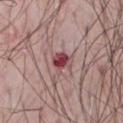Findings:
* follow-up — total-body-photography surveillance lesion; no biopsy
* illumination — white-light
* imaging modality — total-body-photography crop, ~15 mm field of view
* diameter — about 3 mm
* patient — male, aged around 65
* body site — the chest